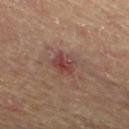Part of a total-body skin-imaging series; this lesion was reviewed on a skin check and was not flagged for biopsy. Automated tile analysis of the lesion measured a border-irregularity index near 2/10, internal color variation of about 6 on a 0–10 scale, and a peripheral color-asymmetry measure near 2. From the right thigh. Captured under cross-polarized illumination. The patient is a male in their mid- to late 60s. A 15 mm crop from a total-body photograph taken for skin-cancer surveillance.